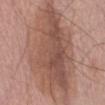Clinical impression:
Part of a total-body skin-imaging series; this lesion was reviewed on a skin check and was not flagged for biopsy.
Context:
A roughly 15 mm field-of-view crop from a total-body skin photograph. The patient is a male aged 63–67. Longest diameter approximately 16 mm. Located on the abdomen. Automated image analysis of the tile measured a lesion color around L≈52 a*≈20 b*≈26 in CIELAB and a lesion-to-skin contrast of about 8 (normalized; higher = more distinct). The software also gave a detector confidence of about 100 out of 100 that the crop contains a lesion.